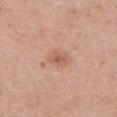biopsy status = total-body-photography surveillance lesion; no biopsy | image source = ~15 mm crop, total-body skin-cancer survey | subject = female, aged 48 to 52 | location = the left upper arm.